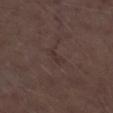The total-body-photography lesion software estimated internal color variation of about 0.5 on a 0–10 scale and peripheral color asymmetry of about 0. The lesion is located on the right lower leg. A 15 mm close-up extracted from a 3D total-body photography capture. Longest diameter approximately 3 mm. The subject is a male about 70 years old. Captured under white-light illumination.